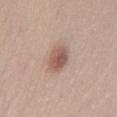<case>
<biopsy_status>not biopsied; imaged during a skin examination</biopsy_status>
<automated_metrics>
  <cielab_L>55</cielab_L>
  <cielab_a>20</cielab_a>
  <cielab_b>25</cielab_b>
  <vs_skin_darker_L>12.0</vs_skin_darker_L>
  <vs_skin_contrast_norm>8.0</vs_skin_contrast_norm>
  <border_irregularity_0_10>2.0</border_irregularity_0_10>
  <color_variation_0_10>4.0</color_variation_0_10>
</automated_metrics>
<lighting>white-light</lighting>
<lesion_size>
  <long_diameter_mm_approx>3.5</long_diameter_mm_approx>
</lesion_size>
<patient>
  <sex>male</sex>
  <age_approx>30</age_approx>
</patient>
<site>mid back</site>
<image>
  <source>total-body photography crop</source>
  <field_of_view_mm>15</field_of_view_mm>
</image>
</case>